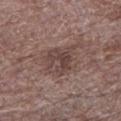Captured during whole-body skin photography for melanoma surveillance; the lesion was not biopsied.
From the left lower leg.
Measured at roughly 5.5 mm in maximum diameter.
A male patient aged 68–72.
Cropped from a total-body skin-imaging series; the visible field is about 15 mm.